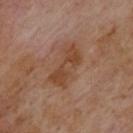The lesion was tiled from a total-body skin photograph and was not biopsied.
From the upper back.
A male patient aged 68–72.
A close-up tile cropped from a whole-body skin photograph, about 15 mm across.
Measured at roughly 5.5 mm in maximum diameter.